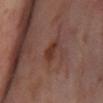<tbp_lesion>
<biopsy_status>not biopsied; imaged during a skin examination</biopsy_status>
<lighting>cross-polarized</lighting>
<site>leg</site>
<image>
  <source>total-body photography crop</source>
  <field_of_view_mm>15</field_of_view_mm>
</image>
<automated_metrics>
  <shape_asymmetry>0.3</shape_asymmetry>
  <cielab_L>35</cielab_L>
  <cielab_a>22</cielab_a>
  <cielab_b>26</cielab_b>
  <vs_skin_contrast_norm>8.0</vs_skin_contrast_norm>
  <border_irregularity_0_10>3.0</border_irregularity_0_10>
</automated_metrics>
<lesion_size>
  <long_diameter_mm_approx>2.5</long_diameter_mm_approx>
</lesion_size>
<patient>
  <sex>female</sex>
  <age_approx>55</age_approx>
</patient>
</tbp_lesion>